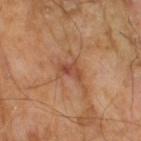biopsy status: no biopsy performed (imaged during a skin exam) | subject: male, in their mid- to late 60s | lesion diameter: ≈3 mm | tile lighting: cross-polarized | acquisition: ~15 mm tile from a whole-body skin photo.